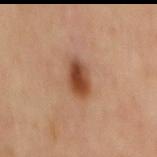body site=the mid back | imaging modality=total-body-photography crop, ~15 mm field of view | tile lighting=cross-polarized illumination | size=~4 mm (longest diameter).